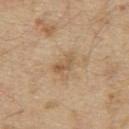notes=no biopsy performed (imaged during a skin exam); subject=male, aged approximately 70; image=~15 mm crop, total-body skin-cancer survey; location=the upper back.